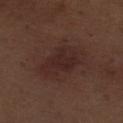Q: Was a biopsy performed?
A: no biopsy performed (imaged during a skin exam)
Q: Who is the patient?
A: male, aged around 70
Q: What lighting was used for the tile?
A: white-light illumination
Q: What kind of image is this?
A: total-body-photography crop, ~15 mm field of view
Q: What is the anatomic site?
A: the left thigh
Q: What is the lesion's diameter?
A: ≈5.5 mm
Q: Automated lesion metrics?
A: a lesion area of about 14 mm², an eccentricity of roughly 0.75, and a symmetry-axis asymmetry near 0.25; a mean CIELAB color near L≈25 a*≈18 b*≈19 and a normalized border contrast of about 7; a border-irregularity index near 3/10, a color-variation rating of about 2.5/10, and radial color variation of about 1